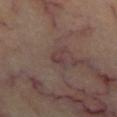Findings:
* lesion size — ~2.5 mm (longest diameter)
* anatomic site — the chest
* imaging modality — ~15 mm crop, total-body skin-cancer survey
* lighting — cross-polarized illumination